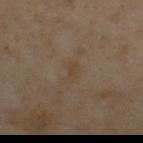Part of a total-body skin-imaging series; this lesion was reviewed on a skin check and was not flagged for biopsy. A male subject, about 55 years old. A 15 mm close-up tile from a total-body photography series done for melanoma screening. Located on the upper back.